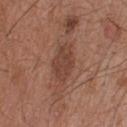<tbp_lesion>
  <automated_metrics>
    <border_irregularity_0_10>2.0</border_irregularity_0_10>
    <color_variation_0_10>2.5</color_variation_0_10>
    <peripheral_color_asymmetry>1.0</peripheral_color_asymmetry>
  </automated_metrics>
  <lighting>white-light</lighting>
  <site>upper back</site>
  <lesion_size>
    <long_diameter_mm_approx>4.0</long_diameter_mm_approx>
  </lesion_size>
  <patient>
    <sex>male</sex>
    <age_approx>45</age_approx>
  </patient>
  <image>
    <source>total-body photography crop</source>
    <field_of_view_mm>15</field_of_view_mm>
  </image>
</tbp_lesion>